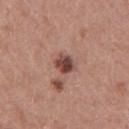Assessment:
No biopsy was performed on this lesion — it was imaged during a full skin examination and was not determined to be concerning.
Background:
Imaged with white-light lighting. On the right upper arm. The total-body-photography lesion software estimated a lesion-to-skin contrast of about 11 (normalized; higher = more distinct). The software also gave an automated nevus-likeness rating near 90 out of 100 and a detector confidence of about 100 out of 100 that the crop contains a lesion. A female patient aged around 40. Cropped from a total-body skin-imaging series; the visible field is about 15 mm. About 2.5 mm across.